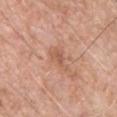The lesion was tiled from a total-body skin photograph and was not biopsied. This image is a 15 mm lesion crop taken from a total-body photograph. Captured under white-light illumination. On the chest. The lesion-visualizer software estimated a border-irregularity rating of about 5/10 and a color-variation rating of about 2/10. And it measured a classifier nevus-likeness of about 0/100 and lesion-presence confidence of about 100/100. A male patient roughly 60 years of age.